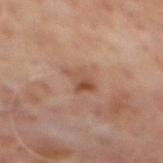{
  "biopsy_status": "not biopsied; imaged during a skin examination"
}